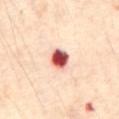* biopsy status: catalogued during a skin exam; not biopsied
* subject: male, roughly 60 years of age
* size: about 2.5 mm
* location: the abdomen
* image-analysis metrics: a footprint of about 5.5 mm², an eccentricity of roughly 0.45, and a symmetry-axis asymmetry near 0.2; an average lesion color of about L≈56 a*≈34 b*≈27 (CIELAB), roughly 27 lightness units darker than nearby skin, and a lesion-to-skin contrast of about 15 (normalized; higher = more distinct); border irregularity of about 1.5 on a 0–10 scale, a color-variation rating of about 9.5/10, and peripheral color asymmetry of about 3
* image source: 15 mm crop, total-body photography